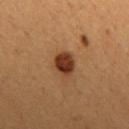image = ~15 mm tile from a whole-body skin photo | patient = male, roughly 45 years of age | diameter = about 3 mm | illumination = cross-polarized | body site = the upper back.